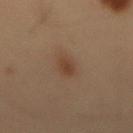Clinical impression: Recorded during total-body skin imaging; not selected for excision or biopsy. Image and clinical context: A male subject aged 53 to 57. A 15 mm crop from a total-body photograph taken for skin-cancer surveillance. Located on the lower back. Captured under cross-polarized illumination.